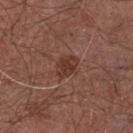follow-up = catalogued during a skin exam; not biopsied
acquisition = 15 mm crop, total-body photography
body site = the front of the torso
lesion diameter = ~3 mm (longest diameter)
subject = male, aged 53–57
automated lesion analysis = a border-irregularity index near 1.5/10, internal color variation of about 3 on a 0–10 scale, and a peripheral color-asymmetry measure near 1; a classifier nevus-likeness of about 55/100